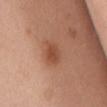follow-up=imaged on a skin check; not biopsied | image-analysis metrics=a within-lesion color-variation index near 3/10; a nevus-likeness score of about 95/100 and a detector confidence of about 100 out of 100 that the crop contains a lesion | patient=female, aged 48–52 | image source=15 mm crop, total-body photography | anatomic site=the front of the torso.